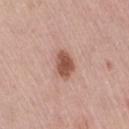biopsy status — no biopsy performed (imaged during a skin exam)
image source — ~15 mm crop, total-body skin-cancer survey
patient — female, in their mid- to late 60s
location — the right thigh